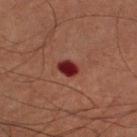workup: total-body-photography surveillance lesion; no biopsy
image source: total-body-photography crop, ~15 mm field of view
anatomic site: the arm
subject: male, aged 58–62
size: ≈2.5 mm
tile lighting: cross-polarized illumination